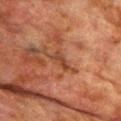follow-up = total-body-photography surveillance lesion; no biopsy
illumination = cross-polarized illumination
size = ~2.5 mm (longest diameter)
subject = male, in their mid- to late 70s
location = the chest
acquisition = 15 mm crop, total-body photography